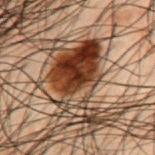The lesion was tiled from a total-body skin photograph and was not biopsied. Captured under cross-polarized illumination. Located on the back. A 15 mm close-up tile from a total-body photography series done for melanoma screening. Automated tile analysis of the lesion measured a footprint of about 33 mm² and a shape eccentricity near 0.85. It also reported a lesion–skin lightness drop of about 14 and a normalized border contrast of about 12.5. It also reported lesion-presence confidence of about 100/100. The subject is a male aged 48 to 52.